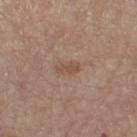{"biopsy_status": "not biopsied; imaged during a skin examination", "patient": {"sex": "female", "age_approx": 65}, "lesion_size": {"long_diameter_mm_approx": 2.5}, "automated_metrics": {"area_mm2_approx": 3.0, "eccentricity": 0.9, "shape_asymmetry": 0.35, "border_irregularity_0_10": 3.5, "color_variation_0_10": 0.5, "peripheral_color_asymmetry": 0.0, "nevus_likeness_0_100": 0, "lesion_detection_confidence_0_100": 100}, "image": {"source": "total-body photography crop", "field_of_view_mm": 15}, "site": "leg"}